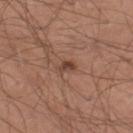This lesion was catalogued during total-body skin photography and was not selected for biopsy. The lesion is on the left thigh. Cropped from a whole-body photographic skin survey; the tile spans about 15 mm. The tile uses white-light illumination. Longest diameter approximately 2.5 mm. A male subject, aged approximately 40. Automated tile analysis of the lesion measured an area of roughly 2.5 mm², an eccentricity of roughly 0.85, and a symmetry-axis asymmetry near 0.25.